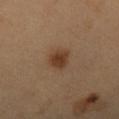No biopsy was performed on this lesion — it was imaged during a full skin examination and was not determined to be concerning. A 15 mm crop from a total-body photograph taken for skin-cancer surveillance. Located on the arm. Automated tile analysis of the lesion measured a lesion-detection confidence of about 100/100. The patient is a male aged approximately 60. Approximately 3 mm at its widest. Captured under cross-polarized illumination.